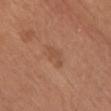This lesion was catalogued during total-body skin photography and was not selected for biopsy. The lesion is located on the chest. The tile uses white-light illumination. Cropped from a whole-body photographic skin survey; the tile spans about 15 mm. Approximately 3.5 mm at its widest. The subject is a female about 65 years old. The lesion-visualizer software estimated an automated nevus-likeness rating near 5 out of 100 and lesion-presence confidence of about 100/100.